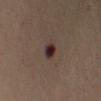The lesion's longest dimension is about 3 mm. Cropped from a whole-body photographic skin survey; the tile spans about 15 mm. The lesion is on the left upper arm. A female patient, aged 43 to 47. The total-body-photography lesion software estimated a lesion area of about 4.5 mm² and a shape-asymmetry score of about 0.2 (0 = symmetric). The analysis additionally found a border-irregularity index near 2/10 and peripheral color asymmetry of about 2.5.